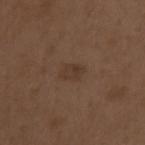Assessment: Captured during whole-body skin photography for melanoma surveillance; the lesion was not biopsied. Clinical summary: A 15 mm crop from a total-body photograph taken for skin-cancer surveillance. The recorded lesion diameter is about 2.5 mm. The lesion is located on the left upper arm. Automated tile analysis of the lesion measured a lesion area of about 4 mm², a shape eccentricity near 0.75, and a symmetry-axis asymmetry near 0.2. The software also gave an average lesion color of about L≈35 a*≈15 b*≈25 (CIELAB), roughly 6 lightness units darker than nearby skin, and a normalized border contrast of about 5.5. It also reported a border-irregularity rating of about 2/10. The analysis additionally found a nevus-likeness score of about 5/100 and a detector confidence of about 100 out of 100 that the crop contains a lesion. A male patient, approximately 70 years of age. The tile uses white-light illumination.